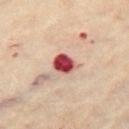The lesion was tiled from a total-body skin photograph and was not biopsied. The recorded lesion diameter is about 3 mm. This is a cross-polarized tile. This image is a 15 mm lesion crop taken from a total-body photograph. A female patient, about 60 years old. The lesion is on the left thigh. The lesion-visualizer software estimated an area of roughly 6 mm², a shape eccentricity near 0.55, and a symmetry-axis asymmetry near 0.2. And it measured about 23 CIELAB-L* units darker than the surrounding skin and a normalized border contrast of about 14.5.